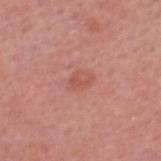Part of a total-body skin-imaging series; this lesion was reviewed on a skin check and was not flagged for biopsy. Captured under white-light illumination. A male patient aged 48 to 52. The lesion is located on the head or neck. This image is a 15 mm lesion crop taken from a total-body photograph.